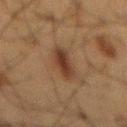Assessment:
No biopsy was performed on this lesion — it was imaged during a full skin examination and was not determined to be concerning.
Acquisition and patient details:
The lesion is located on the mid back. A lesion tile, about 15 mm wide, cut from a 3D total-body photograph. The tile uses cross-polarized illumination. A male subject in their 60s. The lesion's longest dimension is about 5.5 mm.